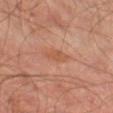Impression:
Recorded during total-body skin imaging; not selected for excision or biopsy.
Background:
Captured under cross-polarized illumination. From the left thigh. Automated tile analysis of the lesion measured a shape-asymmetry score of about 0.25 (0 = symmetric). The analysis additionally found a border-irregularity rating of about 3/10 and a within-lesion color-variation index near 2/10. It also reported a classifier nevus-likeness of about 0/100 and lesion-presence confidence of about 100/100. The patient is a male in their 70s. A roughly 15 mm field-of-view crop from a total-body skin photograph.